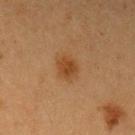follow-up: total-body-photography surveillance lesion; no biopsy | TBP lesion metrics: border irregularity of about 1.5 on a 0–10 scale, internal color variation of about 2.5 on a 0–10 scale, and peripheral color asymmetry of about 1 | image: ~15 mm tile from a whole-body skin photo | diameter: ≈3 mm | patient: female, aged around 40 | lighting: cross-polarized | location: the left upper arm.